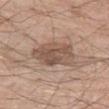Findings:
- notes — no biopsy performed (imaged during a skin exam)
- body site — the left thigh
- imaging modality — 15 mm crop, total-body photography
- tile lighting — white-light
- subject — male, approximately 70 years of age
- diameter — ~5 mm (longest diameter)